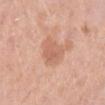Captured during whole-body skin photography for melanoma surveillance; the lesion was not biopsied. Captured under white-light illumination. Approximately 4 mm at its widest. The subject is a female in their mid- to late 50s. The lesion-visualizer software estimated a color-variation rating of about 2.5/10 and peripheral color asymmetry of about 1. A region of skin cropped from a whole-body photographic capture, roughly 15 mm wide. The lesion is located on the leg.